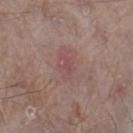lesion_size:
  long_diameter_mm_approx: 2.5
image:
  source: total-body photography crop
  field_of_view_mm: 15
site: left thigh
patient:
  sex: male
  age_approx: 65